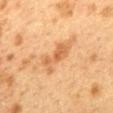Findings:
– workup — total-body-photography surveillance lesion; no biopsy
– image — 15 mm crop, total-body photography
– patient — female, aged 38 to 42
– lighting — cross-polarized illumination
– location — the mid back
– diameter — ~5.5 mm (longest diameter)